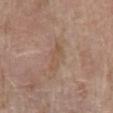{
  "biopsy_status": "not biopsied; imaged during a skin examination",
  "patient": {
    "sex": "female",
    "age_approx": 65
  },
  "lesion_size": {
    "long_diameter_mm_approx": 3.5
  },
  "lighting": "white-light",
  "image": {
    "source": "total-body photography crop",
    "field_of_view_mm": 15
  },
  "site": "left forearm"
}